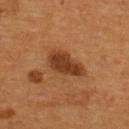Recorded during total-body skin imaging; not selected for excision or biopsy. On the back. A close-up tile cropped from a whole-body skin photograph, about 15 mm across. Measured at roughly 5.5 mm in maximum diameter. This is a cross-polarized tile. A male subject, aged 53 to 57.